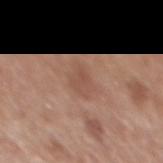patient: male, aged around 70
size: ≈3.5 mm
acquisition: total-body-photography crop, ~15 mm field of view
automated metrics: a lesion area of about 5.5 mm², an outline eccentricity of about 0.75 (0 = round, 1 = elongated), and two-axis asymmetry of about 0.3; a lesion color around L≈51 a*≈21 b*≈28 in CIELAB, roughly 6 lightness units darker than nearby skin, and a normalized lesion–skin contrast near 5; a nevus-likeness score of about 0/100 and a lesion-detection confidence of about 100/100
lighting: white-light
body site: the mid back